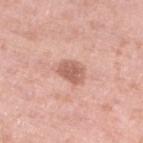Captured during whole-body skin photography for melanoma surveillance; the lesion was not biopsied. Automated image analysis of the tile measured a lesion color around L≈61 a*≈24 b*≈28 in CIELAB, a lesion–skin lightness drop of about 12, and a normalized border contrast of about 7.5. And it measured a nevus-likeness score of about 55/100 and lesion-presence confidence of about 100/100. A female patient, aged 38–42. The tile uses white-light illumination. A 15 mm crop from a total-body photograph taken for skin-cancer surveillance. On the left lower leg.